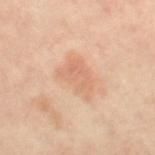Impression:
Captured during whole-body skin photography for melanoma surveillance; the lesion was not biopsied.
Clinical summary:
The lesion is located on the right thigh. This is a cross-polarized tile. Approximately 4.5 mm at its widest. A female patient, about 50 years old. Automated image analysis of the tile measured a border-irregularity index near 3.5/10 and peripheral color asymmetry of about 1.5. It also reported an automated nevus-likeness rating near 0 out of 100. A region of skin cropped from a whole-body photographic capture, roughly 15 mm wide.